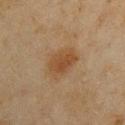Captured under cross-polarized illumination.
Approximately 4 mm at its widest.
The patient is a male aged approximately 45.
Cropped from a total-body skin-imaging series; the visible field is about 15 mm.
On the left upper arm.
The total-body-photography lesion software estimated a mean CIELAB color near L≈37 a*≈16 b*≈29 and a normalized lesion–skin contrast near 7.